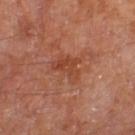  patient:
    sex: male
    age_approx: 70
  lesion_size:
    long_diameter_mm_approx: 3.5
  lighting: cross-polarized
  image:
    source: total-body photography crop
    field_of_view_mm: 15
  site: leg